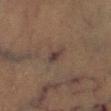Q: Is there a histopathology result?
A: no biopsy performed (imaged during a skin exam)
Q: Patient demographics?
A: male, about 75 years old
Q: Lesion size?
A: ≈2.5 mm
Q: What is the anatomic site?
A: the leg
Q: What kind of image is this?
A: ~15 mm tile from a whole-body skin photo
Q: Illumination type?
A: cross-polarized